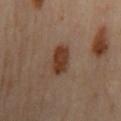This lesion was catalogued during total-body skin photography and was not selected for biopsy.
Approximately 4 mm at its widest.
A male patient, in their mid-60s.
Automated image analysis of the tile measured an eccentricity of roughly 0.85 and a symmetry-axis asymmetry near 0.2. The software also gave a color-variation rating of about 2.5/10 and peripheral color asymmetry of about 1. The software also gave an automated nevus-likeness rating near 100 out of 100 and a detector confidence of about 100 out of 100 that the crop contains a lesion.
Cropped from a total-body skin-imaging series; the visible field is about 15 mm.
The tile uses cross-polarized illumination.
Located on the back.